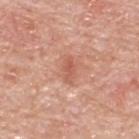Notes:
- workup · total-body-photography surveillance lesion; no biopsy
- automated metrics · about 9 CIELAB-L* units darker than the surrounding skin and a lesion-to-skin contrast of about 6 (normalized; higher = more distinct); border irregularity of about 3 on a 0–10 scale; a nevus-likeness score of about 5/100 and a detector confidence of about 100 out of 100 that the crop contains a lesion
- subject · male, in their 80s
- site · the upper back
- illumination · white-light
- acquisition · 15 mm crop, total-body photography